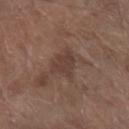notes: total-body-photography surveillance lesion; no biopsy